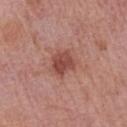notes: catalogued during a skin exam; not biopsied
acquisition: ~15 mm tile from a whole-body skin photo
lesion diameter: ≈3.5 mm
patient: male, about 75 years old
location: the arm
image-analysis metrics: border irregularity of about 3 on a 0–10 scale, internal color variation of about 3 on a 0–10 scale, and a peripheral color-asymmetry measure near 1; an automated nevus-likeness rating near 75 out of 100 and lesion-presence confidence of about 100/100